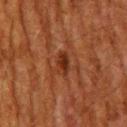  biopsy_status: not biopsied; imaged during a skin examination
  patient:
    sex: male
    age_approx: 60
  automated_metrics:
    eccentricity: 0.8
    shape_asymmetry: 0.2
    cielab_L: 25
    cielab_a: 21
    cielab_b: 27
    vs_skin_darker_L: 8.0
    border_irregularity_0_10: 2.0
    color_variation_0_10: 2.0
    peripheral_color_asymmetry: 0.5
  image:
    source: total-body photography crop
    field_of_view_mm: 15
  site: upper back
  lighting: cross-polarized
  lesion_size:
    long_diameter_mm_approx: 3.0